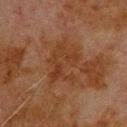Part of a total-body skin-imaging series; this lesion was reviewed on a skin check and was not flagged for biopsy.
A male patient aged approximately 80.
The lesion is located on the upper back.
Imaged with cross-polarized lighting.
This image is a 15 mm lesion crop taken from a total-body photograph.